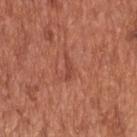  biopsy_status: not biopsied; imaged during a skin examination
  site: back
  lesion_size:
    long_diameter_mm_approx: 2.5
  image:
    source: total-body photography crop
    field_of_view_mm: 15
  patient:
    sex: male
    age_approx: 65
  lighting: white-light